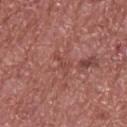notes: no biopsy performed (imaged during a skin exam) | imaging modality: 15 mm crop, total-body photography | patient: male, aged 73–77 | size: about 2.5 mm | location: the upper back | lighting: white-light illumination.